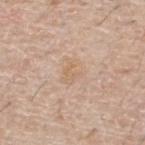Recorded during total-body skin imaging; not selected for excision or biopsy. A male subject aged 53–57. The lesion is located on the upper back. A region of skin cropped from a whole-body photographic capture, roughly 15 mm wide. This is a white-light tile. Longest diameter approximately 3 mm.